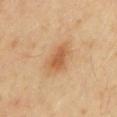workup = total-body-photography surveillance lesion; no biopsy
tile lighting = cross-polarized illumination
anatomic site = the mid back
TBP lesion metrics = a lesion area of about 6.5 mm² and a shape eccentricity near 0.65; a mean CIELAB color near L≈58 a*≈23 b*≈38, roughly 10 lightness units darker than nearby skin, and a normalized lesion–skin contrast near 7; lesion-presence confidence of about 100/100
lesion size = ~3.5 mm (longest diameter)
subject = male, aged approximately 40
imaging modality = ~15 mm crop, total-body skin-cancer survey